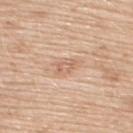Impression: Part of a total-body skin-imaging series; this lesion was reviewed on a skin check and was not flagged for biopsy. Context: A 15 mm close-up tile from a total-body photography series done for melanoma screening. The total-body-photography lesion software estimated border irregularity of about 3 on a 0–10 scale, internal color variation of about 3 on a 0–10 scale, and peripheral color asymmetry of about 1. It also reported a lesion-detection confidence of about 100/100. A female patient, about 55 years old. The lesion is on the upper back. Imaged with white-light lighting.